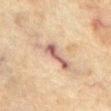biopsy status: catalogued during a skin exam; not biopsied